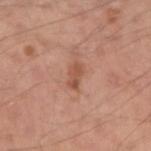Findings:
• biopsy status: imaged on a skin check; not biopsied
• imaging modality: ~15 mm crop, total-body skin-cancer survey
• illumination: white-light
• site: the right upper arm
• lesion diameter: ≈3 mm
• subject: male, approximately 30 years of age